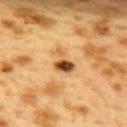| key | value |
|---|---|
| follow-up | catalogued during a skin exam; not biopsied |
| diameter | ≈3 mm |
| site | the upper back |
| subject | female, aged 38–42 |
| tile lighting | cross-polarized |
| image source | ~15 mm tile from a whole-body skin photo |